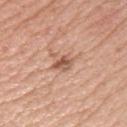No biopsy was performed on this lesion — it was imaged during a full skin examination and was not determined to be concerning. Cropped from a total-body skin-imaging series; the visible field is about 15 mm. Located on the left upper arm. A female patient approximately 55 years of age.